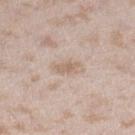biopsy_status: not biopsied; imaged during a skin examination
site: right lower leg
patient:
  sex: female
  age_approx: 25
image:
  source: total-body photography crop
  field_of_view_mm: 15